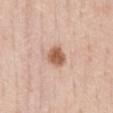Impression:
The lesion was tiled from a total-body skin photograph and was not biopsied.
Context:
Located on the front of the torso. This is a white-light tile. An algorithmic analysis of the crop reported a footprint of about 5.5 mm², a shape eccentricity near 0.55, and two-axis asymmetry of about 0.25. It also reported a lesion color around L≈59 a*≈22 b*≈32 in CIELAB, roughly 14 lightness units darker than nearby skin, and a lesion-to-skin contrast of about 9.5 (normalized; higher = more distinct). It also reported a within-lesion color-variation index near 2.5/10 and a peripheral color-asymmetry measure near 1. The software also gave a detector confidence of about 100 out of 100 that the crop contains a lesion. A male subject about 50 years old. A roughly 15 mm field-of-view crop from a total-body skin photograph.